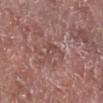location = the right lower leg; subject = male, in their mid-70s; imaging modality = 15 mm crop, total-body photography.